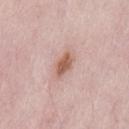follow-up: catalogued during a skin exam; not biopsied
location: the lower back
automated lesion analysis: a footprint of about 5 mm², an outline eccentricity of about 0.75 (0 = round, 1 = elongated), and a shape-asymmetry score of about 0.3 (0 = symmetric)
imaging modality: total-body-photography crop, ~15 mm field of view
patient: female, aged 48–52
lesion diameter: ≈3 mm